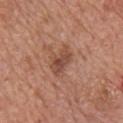follow-up=imaged on a skin check; not biopsied
patient=male, aged 63 to 67
size=≈3.5 mm
body site=the arm
illumination=white-light
image source=~15 mm tile from a whole-body skin photo
automated metrics=a nevus-likeness score of about 25/100 and lesion-presence confidence of about 100/100